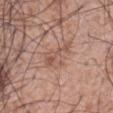• follow-up: total-body-photography surveillance lesion; no biopsy
• lighting: white-light illumination
• subject: male, aged approximately 60
• imaging modality: total-body-photography crop, ~15 mm field of view
• anatomic site: the abdomen